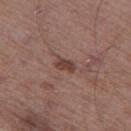• follow-up: no biopsy performed (imaged during a skin exam)
• size: ~3 mm (longest diameter)
• subject: male, aged approximately 65
• image source: total-body-photography crop, ~15 mm field of view
• site: the leg
• TBP lesion metrics: a footprint of about 4 mm² and an outline eccentricity of about 0.8 (0 = round, 1 = elongated); a lesion color around L≈42 a*≈19 b*≈23 in CIELAB and about 9 CIELAB-L* units darker than the surrounding skin; a border-irregularity index near 3/10 and internal color variation of about 1 on a 0–10 scale; an automated nevus-likeness rating near 30 out of 100